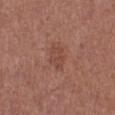Imaged during a routine full-body skin examination; the lesion was not biopsied and no histopathology is available. A female patient roughly 50 years of age. The total-body-photography lesion software estimated a shape eccentricity near 0.7 and a shape-asymmetry score of about 0.3 (0 = symmetric). It also reported a lesion color around L≈45 a*≈23 b*≈27 in CIELAB, a lesion–skin lightness drop of about 6, and a lesion-to-skin contrast of about 5 (normalized; higher = more distinct). And it measured a border-irregularity index near 3/10 and peripheral color asymmetry of about 0.5. The analysis additionally found a classifier nevus-likeness of about 0/100. A 15 mm close-up tile from a total-body photography series done for melanoma screening. This is a white-light tile. The lesion's longest dimension is about 2.5 mm. On the left lower leg.